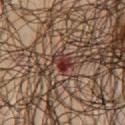Automated image analysis of the tile measured a shape-asymmetry score of about 0.25 (0 = symmetric). Imaged with cross-polarized lighting. From the chest. The patient is a male in their mid- to late 60s. A 15 mm close-up extracted from a 3D total-body photography capture. Longest diameter approximately 3 mm.